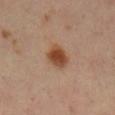No biopsy was performed on this lesion — it was imaged during a full skin examination and was not determined to be concerning.